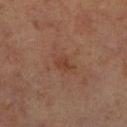Part of a total-body skin-imaging series; this lesion was reviewed on a skin check and was not flagged for biopsy. Captured under cross-polarized illumination. This image is a 15 mm lesion crop taken from a total-body photograph. Automated image analysis of the tile measured a lesion color around L≈31 a*≈18 b*≈24 in CIELAB, about 5 CIELAB-L* units darker than the surrounding skin, and a lesion-to-skin contrast of about 5.5 (normalized; higher = more distinct). A male patient, aged 63–67. From the leg. Approximately 2.5 mm at its widest.